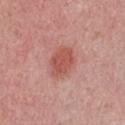Assessment: The lesion was photographed on a routine skin check and not biopsied; there is no pathology result. Context: A female subject aged approximately 25. From the chest. Automated tile analysis of the lesion measured an outline eccentricity of about 0.65 (0 = round, 1 = elongated) and a symmetry-axis asymmetry near 0.15. And it measured a lesion-to-skin contrast of about 7 (normalized; higher = more distinct). The analysis additionally found a color-variation rating of about 2/10 and peripheral color asymmetry of about 0.5. A close-up tile cropped from a whole-body skin photograph, about 15 mm across. The tile uses white-light illumination. About 4 mm across.